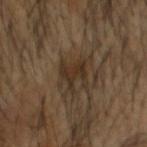Assessment: Captured during whole-body skin photography for melanoma surveillance; the lesion was not biopsied. Clinical summary: Measured at roughly 3 mm in maximum diameter. The lesion is located on the head or neck. Cropped from a total-body skin-imaging series; the visible field is about 15 mm. The subject is a male about 55 years old. Captured under cross-polarized illumination.